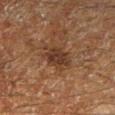<tbp_lesion>
  <biopsy_status>not biopsied; imaged during a skin examination</biopsy_status>
  <image>
    <source>total-body photography crop</source>
    <field_of_view_mm>15</field_of_view_mm>
  </image>
  <patient>
    <sex>male</sex>
    <age_approx>60</age_approx>
  </patient>
  <lesion_size>
    <long_diameter_mm_approx>3.5</long_diameter_mm_approx>
  </lesion_size>
  <lighting>cross-polarized</lighting>
  <site>leg</site>
  <automated_metrics>
    <area_mm2_approx>6.5</area_mm2_approx>
    <eccentricity>0.65</eccentricity>
    <shape_asymmetry>0.2</shape_asymmetry>
    <cielab_L>26</cielab_L>
    <cielab_a>15</cielab_a>
    <cielab_b>22</cielab_b>
    <vs_skin_darker_L>8.0</vs_skin_darker_L>
    <vs_skin_contrast_norm>8.5</vs_skin_contrast_norm>
    <nevus_likeness_0_100>5</nevus_likeness_0_100>
  </automated_metrics>
</tbp_lesion>